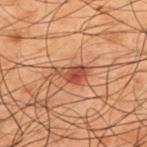The lesion was photographed on a routine skin check and not biopsied; there is no pathology result. The lesion's longest dimension is about 3.5 mm. A male patient approximately 50 years of age. The tile uses cross-polarized illumination. The lesion-visualizer software estimated a nevus-likeness score of about 80/100 and lesion-presence confidence of about 100/100. The lesion is located on the upper back. A roughly 15 mm field-of-view crop from a total-body skin photograph.